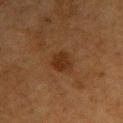Notes:
- image source · ~15 mm crop, total-body skin-cancer survey
- patient · female, in their mid- to late 50s
- anatomic site · the right upper arm